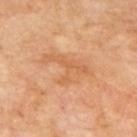Assessment:
The lesion was photographed on a routine skin check and not biopsied; there is no pathology result.
Background:
A male subject, aged around 70. The recorded lesion diameter is about 6 mm. Cropped from a total-body skin-imaging series; the visible field is about 15 mm. Captured under cross-polarized illumination. Located on the mid back.